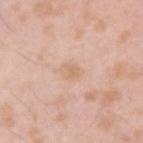Captured during whole-body skin photography for melanoma surveillance; the lesion was not biopsied.
A 15 mm close-up extracted from a 3D total-body photography capture.
A male subject aged approximately 25.
The lesion's longest dimension is about 2.5 mm.
Located on the left upper arm.
An algorithmic analysis of the crop reported a lesion area of about 3.5 mm² and an eccentricity of roughly 0.65. The analysis additionally found a lesion color around L≈68 a*≈19 b*≈32 in CIELAB and a lesion-to-skin contrast of about 5 (normalized; higher = more distinct). And it measured a within-lesion color-variation index near 1.5/10 and radial color variation of about 0.5. It also reported a classifier nevus-likeness of about 0/100 and lesion-presence confidence of about 100/100.